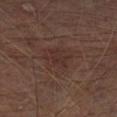* biopsy status: imaged on a skin check; not biopsied
* image source: 15 mm crop, total-body photography
* lesion size: ~2.5 mm (longest diameter)
* anatomic site: the left lower leg
* patient: male, aged around 65
* illumination: cross-polarized illumination